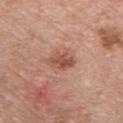Clinical impression:
The lesion was photographed on a routine skin check and not biopsied; there is no pathology result.
Image and clinical context:
A lesion tile, about 15 mm wide, cut from a 3D total-body photograph. The patient is a male aged around 60. From the chest. Imaged with white-light lighting. Approximately 3.5 mm at its widest.